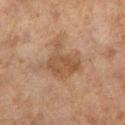Q: Was this lesion biopsied?
A: imaged on a skin check; not biopsied
Q: What is the imaging modality?
A: ~15 mm crop, total-body skin-cancer survey
Q: Lesion size?
A: ~5.5 mm (longest diameter)
Q: What is the anatomic site?
A: the left lower leg
Q: Patient demographics?
A: female, aged approximately 60
Q: What lighting was used for the tile?
A: cross-polarized